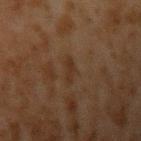Q: Is there a histopathology result?
A: total-body-photography surveillance lesion; no biopsy